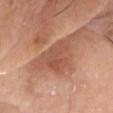Impression:
The lesion was tiled from a total-body skin photograph and was not biopsied.
Clinical summary:
Cropped from a whole-body photographic skin survey; the tile spans about 15 mm. About 5 mm across. This is a white-light tile. A male patient aged approximately 80. Automated tile analysis of the lesion measured an outline eccentricity of about 0.6 (0 = round, 1 = elongated). It also reported an average lesion color of about L≈53 a*≈25 b*≈31 (CIELAB) and roughly 8 lightness units darker than nearby skin. It also reported a classifier nevus-likeness of about 0/100 and a lesion-detection confidence of about 85/100. From the head or neck.